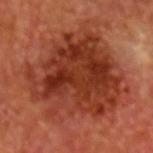Part of a total-body skin-imaging series; this lesion was reviewed on a skin check and was not flagged for biopsy.
A male subject, aged 63 to 67.
The lesion's longest dimension is about 9 mm.
A close-up tile cropped from a whole-body skin photograph, about 15 mm across.
Automated image analysis of the tile measured a lesion color around L≈36 a*≈30 b*≈33 in CIELAB, a lesion–skin lightness drop of about 11, and a normalized lesion–skin contrast near 9. The software also gave a border-irregularity rating of about 5/10 and a peripheral color-asymmetry measure near 2.5.
The lesion is on the head or neck.